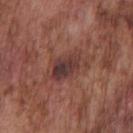The lesion was photographed on a routine skin check and not biopsied; there is no pathology result.
The lesion is located on the front of the torso.
A male patient, about 75 years old.
Captured under white-light illumination.
Cropped from a total-body skin-imaging series; the visible field is about 15 mm.
Automated tile analysis of the lesion measured a footprint of about 10 mm² and a shape-asymmetry score of about 0.35 (0 = symmetric). It also reported an average lesion color of about L≈37 a*≈22 b*≈21 (CIELAB), about 9 CIELAB-L* units darker than the surrounding skin, and a lesion-to-skin contrast of about 9 (normalized; higher = more distinct). And it measured a border-irregularity rating of about 5/10 and a color-variation rating of about 7/10.
Longest diameter approximately 5 mm.